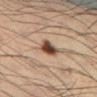Assessment: The lesion was photographed on a routine skin check and not biopsied; there is no pathology result. Clinical summary: A male subject, about 35 years old. A 15 mm close-up tile from a total-body photography series done for melanoma screening. The lesion is located on the left lower leg.